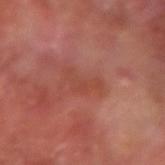Recorded during total-body skin imaging; not selected for excision or biopsy. A 15 mm crop from a total-body photograph taken for skin-cancer surveillance. A male patient roughly 65 years of age. Captured under cross-polarized illumination. The lesion is on the left forearm.